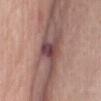Imaged during a routine full-body skin examination; the lesion was not biopsied and no histopathology is available. The lesion is on the chest. Measured at roughly 4.5 mm in maximum diameter. An algorithmic analysis of the crop reported a lesion color around L≈44 a*≈22 b*≈18 in CIELAB, a lesion–skin lightness drop of about 14, and a normalized lesion–skin contrast near 11. And it measured an automated nevus-likeness rating near 25 out of 100 and a detector confidence of about 95 out of 100 that the crop contains a lesion. This is a white-light tile. A male patient approximately 75 years of age. A 15 mm crop from a total-body photograph taken for skin-cancer surveillance.